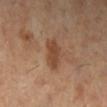notes: imaged on a skin check; not biopsied
size: ≈3.5 mm
body site: the left lower leg
image: ~15 mm crop, total-body skin-cancer survey
illumination: cross-polarized
subject: female, about 60 years old